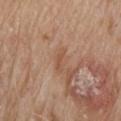Part of a total-body skin-imaging series; this lesion was reviewed on a skin check and was not flagged for biopsy. On the head or neck. This is a white-light tile. The recorded lesion diameter is about 2.5 mm. A lesion tile, about 15 mm wide, cut from a 3D total-body photograph. The patient is a male aged 78 to 82.